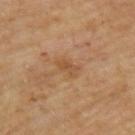This lesion was catalogued during total-body skin photography and was not selected for biopsy.
Captured under cross-polarized illumination.
Approximately 3 mm at its widest.
A male patient aged 68 to 72.
This image is a 15 mm lesion crop taken from a total-body photograph.
On the upper back.